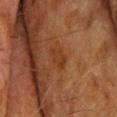follow-up — catalogued during a skin exam; not biopsied | patient — male, aged 73–77 | imaging modality — total-body-photography crop, ~15 mm field of view | lighting — cross-polarized illumination | lesion size — ~3 mm (longest diameter) | body site — the upper back | TBP lesion metrics — a lesion area of about 4 mm², an outline eccentricity of about 0.85 (0 = round, 1 = elongated), and a symmetry-axis asymmetry near 0.3; a lesion–skin lightness drop of about 5 and a lesion-to-skin contrast of about 5.5 (normalized; higher = more distinct); border irregularity of about 3.5 on a 0–10 scale and radial color variation of about 0.5.